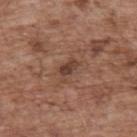The lesion was photographed on a routine skin check and not biopsied; there is no pathology result. An algorithmic analysis of the crop reported a mean CIELAB color near L≈40 a*≈20 b*≈25, a lesion–skin lightness drop of about 10, and a lesion-to-skin contrast of about 8 (normalized; higher = more distinct). And it measured border irregularity of about 4 on a 0–10 scale. The analysis additionally found a classifier nevus-likeness of about 0/100 and lesion-presence confidence of about 100/100. A roughly 15 mm field-of-view crop from a total-body skin photograph. A male subject aged around 70. The lesion's longest dimension is about 3 mm. Imaged with white-light lighting. Located on the back.